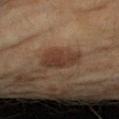This lesion was catalogued during total-body skin photography and was not selected for biopsy. A female patient, aged approximately 60. This is a cross-polarized tile. A 15 mm close-up tile from a total-body photography series done for melanoma screening. The recorded lesion diameter is about 4.5 mm. On the right upper arm.